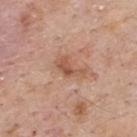biopsy status: imaged on a skin check; not biopsied
location: the upper back
patient: male, aged 58 to 62
tile lighting: white-light illumination
size: about 3.5 mm
image-analysis metrics: a lesion area of about 4.5 mm² and a symmetry-axis asymmetry near 0.5; a border-irregularity index near 5/10 and peripheral color asymmetry of about 1
imaging modality: ~15 mm tile from a whole-body skin photo